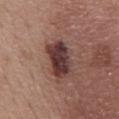Notes:
• body site · the chest
• lighting · white-light
• patient · female, roughly 45 years of age
• image source · total-body-photography crop, ~15 mm field of view
• size · ~5 mm (longest diameter)